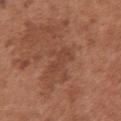Imaged during a routine full-body skin examination; the lesion was not biopsied and no histopathology is available. Automated image analysis of the tile measured an outline eccentricity of about 0.9 (0 = round, 1 = elongated) and two-axis asymmetry of about 0.3. The analysis additionally found a normalized border contrast of about 4.5. The analysis additionally found a nevus-likeness score of about 0/100 and a lesion-detection confidence of about 100/100. Cropped from a whole-body photographic skin survey; the tile spans about 15 mm. From the chest. About 4 mm across. The tile uses white-light illumination. A female subject roughly 65 years of age.